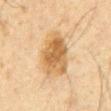Q: Where on the body is the lesion?
A: the chest
Q: What are the patient's age and sex?
A: male, in their 60s
Q: How was this image acquired?
A: ~15 mm tile from a whole-body skin photo